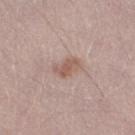Part of a total-body skin-imaging series; this lesion was reviewed on a skin check and was not flagged for biopsy. This image is a 15 mm lesion crop taken from a total-body photograph. The recorded lesion diameter is about 3 mm. A male subject, roughly 30 years of age. This is a white-light tile. Automated image analysis of the tile measured a footprint of about 5.5 mm², an outline eccentricity of about 0.8 (0 = round, 1 = elongated), and a symmetry-axis asymmetry near 0.2. And it measured an average lesion color of about L≈57 a*≈18 b*≈25 (CIELAB), about 9 CIELAB-L* units darker than the surrounding skin, and a lesion-to-skin contrast of about 6.5 (normalized; higher = more distinct). The software also gave an automated nevus-likeness rating near 55 out of 100.